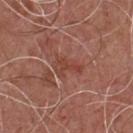Impression: The lesion was tiled from a total-body skin photograph and was not biopsied. Image and clinical context: Approximately 3.5 mm at its widest. The lesion is on the chest. A close-up tile cropped from a whole-body skin photograph, about 15 mm across. The tile uses white-light illumination. A male patient, aged around 55.